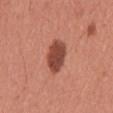Impression:
This lesion was catalogued during total-body skin photography and was not selected for biopsy.
Acquisition and patient details:
Approximately 4 mm at its widest. The subject is a male roughly 45 years of age. A lesion tile, about 15 mm wide, cut from a 3D total-body photograph. Automated image analysis of the tile measured a lesion area of about 9.5 mm², a shape eccentricity near 0.75, and a symmetry-axis asymmetry near 0.15. The analysis additionally found a nevus-likeness score of about 95/100. The lesion is located on the abdomen.